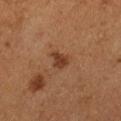This lesion was catalogued during total-body skin photography and was not selected for biopsy.
A female subject, aged around 50.
The lesion's longest dimension is about 3 mm.
This image is a 15 mm lesion crop taken from a total-body photograph.
Imaged with cross-polarized lighting.
The lesion is on the left thigh.